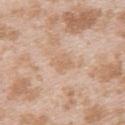<record>
<biopsy_status>not biopsied; imaged during a skin examination</biopsy_status>
<patient>
  <sex>female</sex>
  <age_approx>25</age_approx>
</patient>
<lesion_size>
  <long_diameter_mm_approx>2.5</long_diameter_mm_approx>
</lesion_size>
<lighting>white-light</lighting>
<site>upper back</site>
<image>
  <source>total-body photography crop</source>
  <field_of_view_mm>15</field_of_view_mm>
</image>
</record>